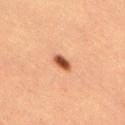biopsy status — total-body-photography surveillance lesion; no biopsy
site — the leg
size — about 2.5 mm
subject — female, aged 38 to 42
lighting — cross-polarized
image — 15 mm crop, total-body photography